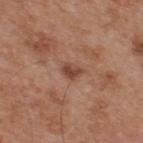Impression:
Recorded during total-body skin imaging; not selected for excision or biopsy.
Context:
The lesion is on the upper back. Measured at roughly 2.5 mm in maximum diameter. A 15 mm crop from a total-body photograph taken for skin-cancer surveillance. The patient is a male roughly 55 years of age. The tile uses white-light illumination.